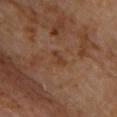Findings:
- workup — catalogued during a skin exam; not biopsied
- imaging modality — ~15 mm crop, total-body skin-cancer survey
- subject — male, aged around 60
- location — the front of the torso
- TBP lesion metrics — a lesion area of about 3 mm², a shape eccentricity near 0.85, and a shape-asymmetry score of about 0.4 (0 = symmetric); an average lesion color of about L≈37 a*≈20 b*≈30 (CIELAB)
- lesion size — ~2.5 mm (longest diameter)